A male patient, about 60 years old · a close-up tile cropped from a whole-body skin photograph, about 15 mm across: 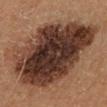Case summary:
* size: about 10.5 mm
* image-analysis metrics: a border-irregularity index near 3/10, a within-lesion color-variation index near 7.5/10, and a peripheral color-asymmetry measure near 2.5
* lighting: cross-polarized
* histopathology: a dysplastic (Clark) nevus